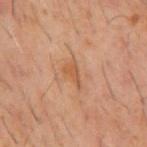Q: Was this lesion biopsied?
A: total-body-photography surveillance lesion; no biopsy
Q: What is the anatomic site?
A: the mid back
Q: What are the patient's age and sex?
A: male, about 60 years old
Q: What kind of image is this?
A: ~15 mm tile from a whole-body skin photo